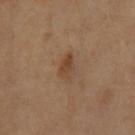| feature | finding |
|---|---|
| workup | total-body-photography surveillance lesion; no biopsy |
| body site | the right thigh |
| subject | female, aged 53 to 57 |
| TBP lesion metrics | a shape-asymmetry score of about 0.3 (0 = symmetric); a mean CIELAB color near L≈44 a*≈18 b*≈31, about 8 CIELAB-L* units darker than the surrounding skin, and a normalized border contrast of about 6.5; a nevus-likeness score of about 25/100 and a detector confidence of about 100 out of 100 that the crop contains a lesion |
| tile lighting | cross-polarized |
| image | total-body-photography crop, ~15 mm field of view |